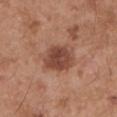subject: male, roughly 55 years of age
tile lighting: white-light
automated lesion analysis: an area of roughly 11 mm², an eccentricity of roughly 0.6, and a shape-asymmetry score of about 0.2 (0 = symmetric); roughly 12 lightness units darker than nearby skin and a lesion-to-skin contrast of about 9 (normalized; higher = more distinct); a color-variation rating of about 3.5/10 and peripheral color asymmetry of about 1
size: ~4 mm (longest diameter)
anatomic site: the arm
image source: ~15 mm tile from a whole-body skin photo